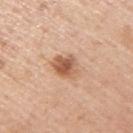biopsy status — total-body-photography surveillance lesion; no biopsy | location — the right upper arm | illumination — white-light | subject — male, approximately 75 years of age | image source — 15 mm crop, total-body photography | automated lesion analysis — an average lesion color of about L≈58 a*≈22 b*≈33 (CIELAB), a lesion–skin lightness drop of about 13, and a normalized border contrast of about 8.5; a border-irregularity rating of about 2.5/10, a color-variation rating of about 5/10, and peripheral color asymmetry of about 1.5; a classifier nevus-likeness of about 95/100 and a detector confidence of about 100 out of 100 that the crop contains a lesion | diameter — ~3.5 mm (longest diameter).